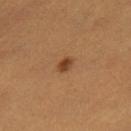The lesion was tiled from a total-body skin photograph and was not biopsied. Imaged with cross-polarized lighting. From the leg. Cropped from a whole-body photographic skin survey; the tile spans about 15 mm. Measured at roughly 2 mm in maximum diameter. A female subject, aged around 40.